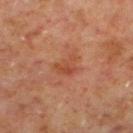Clinical impression:
Captured during whole-body skin photography for melanoma surveillance; the lesion was not biopsied.
Context:
Captured under cross-polarized illumination. From the left lower leg. Cropped from a total-body skin-imaging series; the visible field is about 15 mm. Approximately 3 mm at its widest. The lesion-visualizer software estimated an area of roughly 5 mm², a shape eccentricity near 0.55, and a shape-asymmetry score of about 0.45 (0 = symmetric). It also reported a within-lesion color-variation index near 3.5/10 and a peripheral color-asymmetry measure near 1. The software also gave a classifier nevus-likeness of about 0/100. A male patient, roughly 60 years of age.